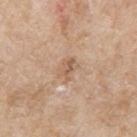Notes:
- workup: no biopsy performed (imaged during a skin exam)
- tile lighting: white-light illumination
- body site: the arm
- lesion size: ~2.5 mm (longest diameter)
- subject: male, aged 63–67
- acquisition: ~15 mm tile from a whole-body skin photo